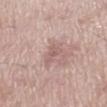Q: Was a biopsy performed?
A: imaged on a skin check; not biopsied